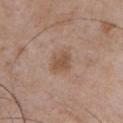  biopsy_status: not biopsied; imaged during a skin examination
  patient:
    sex: male
    age_approx: 50
  lesion_size:
    long_diameter_mm_approx: 2.5
  site: chest
  automated_metrics:
    cielab_L: 51
    cielab_a: 18
    cielab_b: 29
    vs_skin_darker_L: 9.0
    vs_skin_contrast_norm: 7.0
    border_irregularity_0_10: 2.0
    peripheral_color_asymmetry: 0.5
  image:
    source: total-body photography crop
    field_of_view_mm: 15